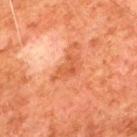{"biopsy_status": "not biopsied; imaged during a skin examination", "patient": {"sex": "male", "age_approx": 80}, "automated_metrics": {"cielab_L": 45, "cielab_a": 27, "cielab_b": 35, "vs_skin_darker_L": 8.0, "vs_skin_contrast_norm": 6.5, "border_irregularity_0_10": 4.5, "peripheral_color_asymmetry": 0.5}, "lesion_size": {"long_diameter_mm_approx": 4.0}, "image": {"source": "total-body photography crop", "field_of_view_mm": 15}, "site": "upper back", "lighting": "cross-polarized"}